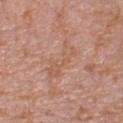{
  "biopsy_status": "not biopsied; imaged during a skin examination",
  "automated_metrics": {
    "eccentricity": 0.95,
    "shape_asymmetry": 0.45,
    "cielab_L": 57,
    "cielab_a": 21,
    "cielab_b": 31,
    "lesion_detection_confidence_0_100": 95
  },
  "site": "right lower leg",
  "lesion_size": {
    "long_diameter_mm_approx": 5.0
  },
  "lighting": "white-light",
  "image": {
    "source": "total-body photography crop",
    "field_of_view_mm": 15
  },
  "patient": {
    "sex": "female",
    "age_approx": 40
  }
}